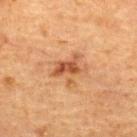Located on the upper back. The lesion's longest dimension is about 4 mm. Imaged with cross-polarized lighting. A 15 mm close-up extracted from a 3D total-body photography capture. A female subject, aged approximately 70.